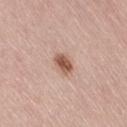{"biopsy_status": "not biopsied; imaged during a skin examination", "site": "right thigh", "automated_metrics": {"cielab_L": 56, "cielab_a": 21, "cielab_b": 28, "vs_skin_contrast_norm": 9.5, "border_irregularity_0_10": 1.5, "color_variation_0_10": 2.5, "peripheral_color_asymmetry": 1.0, "lesion_detection_confidence_0_100": 100}, "patient": {"sex": "female", "age_approx": 65}, "lesion_size": {"long_diameter_mm_approx": 2.5}, "image": {"source": "total-body photography crop", "field_of_view_mm": 15}}